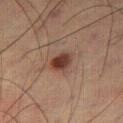Imaged during a routine full-body skin examination; the lesion was not biopsied and no histopathology is available. The total-body-photography lesion software estimated a lesion area of about 5.5 mm², an eccentricity of roughly 0.6, and a shape-asymmetry score of about 0.2 (0 = symmetric). And it measured an average lesion color of about L≈34 a*≈19 b*≈23 (CIELAB), roughly 11 lightness units darker than nearby skin, and a normalized border contrast of about 10.5. And it measured a border-irregularity rating of about 1.5/10, a color-variation rating of about 4.5/10, and a peripheral color-asymmetry measure near 1.5. The analysis additionally found an automated nevus-likeness rating near 100 out of 100 and lesion-presence confidence of about 100/100. The recorded lesion diameter is about 3 mm. Captured under cross-polarized illumination. A lesion tile, about 15 mm wide, cut from a 3D total-body photograph. A male patient in their 60s.